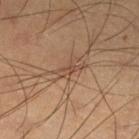{"biopsy_status": "not biopsied; imaged during a skin examination", "automated_metrics": {"area_mm2_approx": 3.0, "eccentricity": 0.95, "shape_asymmetry": 0.4, "nevus_likeness_0_100": 0, "lesion_detection_confidence_0_100": 60}, "lesion_size": {"long_diameter_mm_approx": 3.0}, "lighting": "cross-polarized", "image": {"source": "total-body photography crop", "field_of_view_mm": 15}, "site": "left lower leg", "patient": {"sex": "male", "age_approx": 35}}